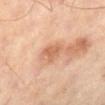Impression:
The lesion was tiled from a total-body skin photograph and was not biopsied.
Image and clinical context:
A male patient in their mid- to late 60s. Captured under cross-polarized illumination. Automated tile analysis of the lesion measured an average lesion color of about L≈58 a*≈22 b*≈32 (CIELAB), roughly 10 lightness units darker than nearby skin, and a normalized border contrast of about 6.5. And it measured a color-variation rating of about 2/10. Located on the right lower leg. A roughly 15 mm field-of-view crop from a total-body skin photograph.